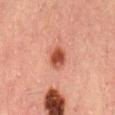Q: Was this lesion biopsied?
A: no biopsy performed (imaged during a skin exam)
Q: Who is the patient?
A: male, aged 48 to 52
Q: Illumination type?
A: cross-polarized illumination
Q: How large is the lesion?
A: about 3 mm
Q: What is the imaging modality?
A: 15 mm crop, total-body photography
Q: What is the anatomic site?
A: the mid back